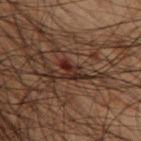<tbp_lesion>
<biopsy_status>not biopsied; imaged during a skin examination</biopsy_status>
<site>left thigh</site>
<image>
  <source>total-body photography crop</source>
  <field_of_view_mm>15</field_of_view_mm>
</image>
<lesion_size>
  <long_diameter_mm_approx>2.5</long_diameter_mm_approx>
</lesion_size>
<lighting>cross-polarized</lighting>
<patient>
  <sex>male</sex>
  <age_approx>50</age_approx>
</patient>
</tbp_lesion>